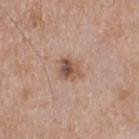Clinical impression: No biopsy was performed on this lesion — it was imaged during a full skin examination and was not determined to be concerning. Clinical summary: A 15 mm close-up extracted from a 3D total-body photography capture. The recorded lesion diameter is about 3 mm. Automated tile analysis of the lesion measured a mean CIELAB color near L≈53 a*≈18 b*≈27, about 12 CIELAB-L* units darker than the surrounding skin, and a normalized border contrast of about 8. It also reported a lesion-detection confidence of about 100/100. The tile uses white-light illumination. The patient is a male aged 53–57.